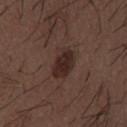Impression: This lesion was catalogued during total-body skin photography and was not selected for biopsy. Image and clinical context: Located on the mid back. The subject is a male aged 48 to 52. Measured at roughly 4 mm in maximum diameter. A 15 mm close-up extracted from a 3D total-body photography capture. Imaged with white-light lighting.